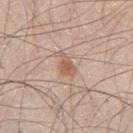Q: Was this lesion biopsied?
A: total-body-photography surveillance lesion; no biopsy
Q: What is the imaging modality?
A: ~15 mm crop, total-body skin-cancer survey
Q: What is the lesion's diameter?
A: ~3 mm (longest diameter)
Q: How was the tile lit?
A: white-light
Q: Where on the body is the lesion?
A: the left thigh
Q: Who is the patient?
A: male, in their 60s